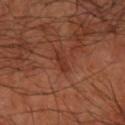Recorded during total-body skin imaging; not selected for excision or biopsy. The lesion is located on the right forearm. A male patient roughly 65 years of age. A 15 mm close-up tile from a total-body photography series done for melanoma screening.